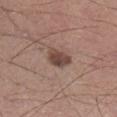Part of a total-body skin-imaging series; this lesion was reviewed on a skin check and was not flagged for biopsy. On the right lower leg. A 15 mm close-up extracted from a 3D total-body photography capture. The patient is a male aged around 55.